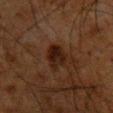No biopsy was performed on this lesion — it was imaged during a full skin examination and was not determined to be concerning. A 15 mm close-up extracted from a 3D total-body photography capture. The lesion is on the chest. A male subject, about 65 years old.